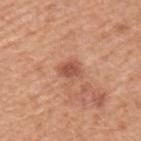Q: What kind of image is this?
A: ~15 mm crop, total-body skin-cancer survey
Q: Patient demographics?
A: male, aged 48 to 52
Q: How was the tile lit?
A: white-light illumination
Q: Lesion location?
A: the arm
Q: How large is the lesion?
A: ~3 mm (longest diameter)